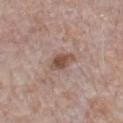Captured during whole-body skin photography for melanoma surveillance; the lesion was not biopsied.
Captured under white-light illumination.
A close-up tile cropped from a whole-body skin photograph, about 15 mm across.
On the chest.
A male subject, aged 68 to 72.
Automated image analysis of the tile measured a lesion color around L≈50 a*≈19 b*≈25 in CIELAB, about 11 CIELAB-L* units darker than the surrounding skin, and a lesion-to-skin contrast of about 8.5 (normalized; higher = more distinct). And it measured an automated nevus-likeness rating near 85 out of 100 and a lesion-detection confidence of about 100/100.
About 3.5 mm across.